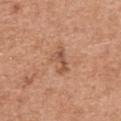Q: Is there a histopathology result?
A: no biopsy performed (imaged during a skin exam)
Q: Lesion size?
A: about 3.5 mm
Q: Where on the body is the lesion?
A: the back
Q: What kind of image is this?
A: 15 mm crop, total-body photography
Q: What are the patient's age and sex?
A: female, approximately 60 years of age